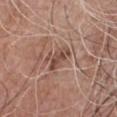| key | value |
|---|---|
| biopsy status | no biopsy performed (imaged during a skin exam) |
| imaging modality | ~15 mm crop, total-body skin-cancer survey |
| subject | male, about 65 years old |
| size | ~3.5 mm (longest diameter) |
| automated lesion analysis | a nevus-likeness score of about 0/100 and a lesion-detection confidence of about 95/100 |
| body site | the chest |